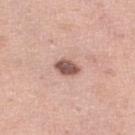biopsy status = no biopsy performed (imaged during a skin exam)
lesion diameter = about 3 mm
tile lighting = white-light
patient = female, aged 38 to 42
site = the right lower leg
imaging modality = ~15 mm tile from a whole-body skin photo
automated metrics = an area of roughly 4.5 mm² and a shape eccentricity near 0.8; a lesion color around L≈54 a*≈21 b*≈24 in CIELAB; internal color variation of about 2 on a 0–10 scale and peripheral color asymmetry of about 0.5; a nevus-likeness score of about 70/100 and a lesion-detection confidence of about 100/100